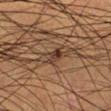The lesion was photographed on a routine skin check and not biopsied; there is no pathology result.
This image is a 15 mm lesion crop taken from a total-body photograph.
The total-body-photography lesion software estimated a footprint of about 2.5 mm² and an eccentricity of roughly 0.85.
The lesion's longest dimension is about 2.5 mm.
A male patient aged around 50.
Located on the left lower leg.